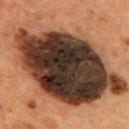Case summary:
• workup · total-body-photography surveillance lesion; no biopsy